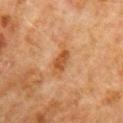notes — catalogued during a skin exam; not biopsied | site — the right upper arm | image — ~15 mm tile from a whole-body skin photo | diameter — about 3 mm | subject — male, aged approximately 80 | TBP lesion metrics — an average lesion color of about L≈40 a*≈21 b*≈34 (CIELAB) and a lesion-to-skin contrast of about 8 (normalized; higher = more distinct); an automated nevus-likeness rating near 15 out of 100 and a lesion-detection confidence of about 100/100.